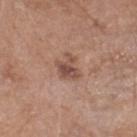notes: catalogued during a skin exam; not biopsied
illumination: white-light illumination
patient: male, aged around 55
image: total-body-photography crop, ~15 mm field of view
size: ≈3.5 mm
body site: the head or neck